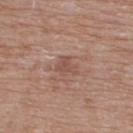Assessment:
The lesion was tiled from a total-body skin photograph and was not biopsied.
Clinical summary:
A male patient, aged around 75. The recorded lesion diameter is about 2.5 mm. A roughly 15 mm field-of-view crop from a total-body skin photograph. Automated tile analysis of the lesion measured border irregularity of about 5 on a 0–10 scale, a color-variation rating of about 1.5/10, and a peripheral color-asymmetry measure near 0.5. It also reported an automated nevus-likeness rating near 0 out of 100 and a detector confidence of about 100 out of 100 that the crop contains a lesion. The lesion is on the upper back. Imaged with white-light lighting.